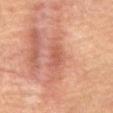follow-up: total-body-photography surveillance lesion; no biopsy
site: the abdomen
tile lighting: cross-polarized illumination
imaging modality: ~15 mm crop, total-body skin-cancer survey
subject: male, roughly 55 years of age
lesion diameter: about 3 mm